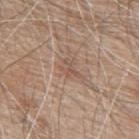Imaged during a routine full-body skin examination; the lesion was not biopsied and no histopathology is available. The recorded lesion diameter is about 3 mm. From the upper back. The patient is a male about 80 years old. Cropped from a whole-body photographic skin survey; the tile spans about 15 mm. The tile uses white-light illumination.